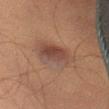Imaged during a routine full-body skin examination; the lesion was not biopsied and no histopathology is available.
An algorithmic analysis of the crop reported a border-irregularity rating of about 2.5/10, internal color variation of about 4.5 on a 0–10 scale, and radial color variation of about 1.5.
A male subject, about 70 years old.
A close-up tile cropped from a whole-body skin photograph, about 15 mm across.
Captured under cross-polarized illumination.
About 5.5 mm across.
The lesion is on the right thigh.